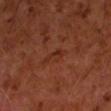Captured during whole-body skin photography for melanoma surveillance; the lesion was not biopsied. A region of skin cropped from a whole-body photographic capture, roughly 15 mm wide. The lesion's longest dimension is about 2.5 mm. Captured under cross-polarized illumination. The lesion is on the left forearm. A male subject aged 58 to 62.